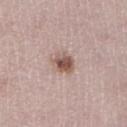<case>
<biopsy_status>not biopsied; imaged during a skin examination</biopsy_status>
<site>left lower leg</site>
<patient>
  <sex>female</sex>
  <age_approx>50</age_approx>
</patient>
<image>
  <source>total-body photography crop</source>
  <field_of_view_mm>15</field_of_view_mm>
</image>
</case>